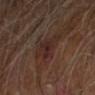biopsy status: catalogued during a skin exam; not biopsied
image-analysis metrics: a footprint of about 6.5 mm², an outline eccentricity of about 0.6 (0 = round, 1 = elongated), and a symmetry-axis asymmetry near 0.45; a nevus-likeness score of about 0/100 and lesion-presence confidence of about 100/100
patient: male, approximately 60 years of age
lighting: cross-polarized illumination
acquisition: total-body-photography crop, ~15 mm field of view
body site: the left arm
lesion diameter: about 3.5 mm